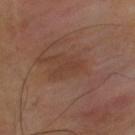• location · the upper back
• illumination · cross-polarized
• image source · total-body-photography crop, ~15 mm field of view
• subject · male, in their 40s
• TBP lesion metrics · a lesion area of about 2.5 mm² and two-axis asymmetry of about 0.5